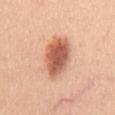{
  "biopsy_status": "not biopsied; imaged during a skin examination",
  "site": "chest",
  "lesion_size": {
    "long_diameter_mm_approx": 6.0
  },
  "image": {
    "source": "total-body photography crop",
    "field_of_view_mm": 15
  },
  "patient": {
    "sex": "male",
    "age_approx": 45
  }
}